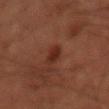workup — no biopsy performed (imaged during a skin exam)
acquisition — ~15 mm crop, total-body skin-cancer survey
lesion size — ≈2.5 mm
subject — male, aged 68–72
anatomic site — the right forearm